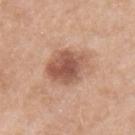A female subject, approximately 55 years of age.
The lesion is on the left upper arm.
A region of skin cropped from a whole-body photographic capture, roughly 15 mm wide.
Approximately 4.5 mm at its widest.